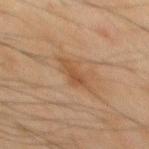Assessment:
The lesion was tiled from a total-body skin photograph and was not biopsied.
Background:
The lesion is located on the mid back. A male patient, aged 43 to 47. Cropped from a whole-body photographic skin survey; the tile spans about 15 mm. The tile uses cross-polarized illumination. Approximately 3.5 mm at its widest.